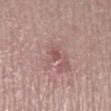notes = no biopsy performed (imaged during a skin exam)
automated metrics = a footprint of about 1.5 mm², an eccentricity of roughly 0.65, and a symmetry-axis asymmetry near 0.3; a border-irregularity index near 2.5/10, a within-lesion color-variation index near 0/10, and peripheral color asymmetry of about 0
imaging modality = ~15 mm crop, total-body skin-cancer survey
patient = female, in their 50s
body site = the right lower leg
lesion size = about 1.5 mm
lighting = white-light illumination